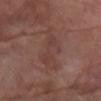{
  "biopsy_status": "not biopsied; imaged during a skin examination",
  "patient": {
    "sex": "female",
    "age_approx": 70
  },
  "image": {
    "source": "total-body photography crop",
    "field_of_view_mm": 15
  },
  "lighting": "white-light",
  "lesion_size": {
    "long_diameter_mm_approx": 6.5
  },
  "site": "right forearm"
}